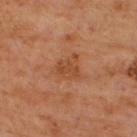Captured during whole-body skin photography for melanoma surveillance; the lesion was not biopsied.
This is a cross-polarized tile.
The lesion is on the upper back.
A 15 mm close-up tile from a total-body photography series done for melanoma screening.
The total-body-photography lesion software estimated an outline eccentricity of about 0.45 (0 = round, 1 = elongated) and a shape-asymmetry score of about 0.35 (0 = symmetric).
A male patient aged around 65.
Longest diameter approximately 3 mm.